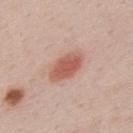Case summary:
• follow-up · total-body-photography surveillance lesion; no biopsy
• size · about 4.5 mm
• automated lesion analysis · a border-irregularity rating of about 2/10 and peripheral color asymmetry of about 1; an automated nevus-likeness rating near 100 out of 100 and lesion-presence confidence of about 100/100
• illumination · white-light
• patient · male, aged 48–52
• image source · total-body-photography crop, ~15 mm field of view
• site · the mid back